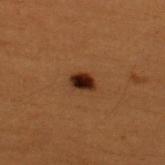Q: Was a biopsy performed?
A: no biopsy performed (imaged during a skin exam)
Q: Automated lesion metrics?
A: a footprint of about 4 mm²
Q: What lighting was used for the tile?
A: cross-polarized illumination
Q: Who is the patient?
A: male, roughly 50 years of age
Q: Where on the body is the lesion?
A: the upper back
Q: How large is the lesion?
A: ~2.5 mm (longest diameter)
Q: How was this image acquired?
A: ~15 mm tile from a whole-body skin photo